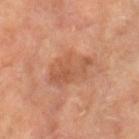No biopsy was performed on this lesion — it was imaged during a full skin examination and was not determined to be concerning. A 15 mm close-up tile from a total-body photography series done for melanoma screening. On the left thigh. Automated image analysis of the tile measured a lesion area of about 14 mm², an outline eccentricity of about 0.7 (0 = round, 1 = elongated), and two-axis asymmetry of about 0.2. This is a cross-polarized tile. A female patient aged 68–72. The recorded lesion diameter is about 5.5 mm.